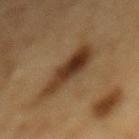  biopsy_status: not biopsied; imaged during a skin examination
  site: mid back
  automated_metrics:
    peripheral_color_asymmetry: 2.0
  image:
    source: total-body photography crop
    field_of_view_mm: 15
  patient:
    sex: male
    age_approx: 85+